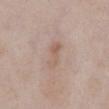Findings:
• notes · no biopsy performed (imaged during a skin exam)
• subject · male, aged approximately 55
• body site · the front of the torso
• image · 15 mm crop, total-body photography